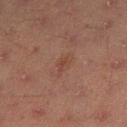No biopsy was performed on this lesion — it was imaged during a full skin examination and was not determined to be concerning.